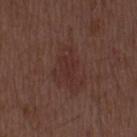Findings:
• follow-up — catalogued during a skin exam; not biopsied
• image source — 15 mm crop, total-body photography
• patient — male, aged around 50
• diameter — ≈3 mm
• tile lighting — white-light